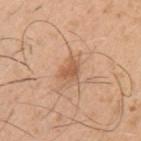Captured during whole-body skin photography for melanoma surveillance; the lesion was not biopsied. The patient is a male roughly 50 years of age. Imaged with white-light lighting. A lesion tile, about 15 mm wide, cut from a 3D total-body photograph. The recorded lesion diameter is about 2.5 mm. On the left upper arm.